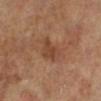Part of a total-body skin-imaging series; this lesion was reviewed on a skin check and was not flagged for biopsy. A 15 mm crop from a total-body photograph taken for skin-cancer surveillance. A female patient aged 63–67. Imaged with cross-polarized lighting. The lesion-visualizer software estimated a lesion area of about 6.5 mm² and a symmetry-axis asymmetry near 0.3. The analysis additionally found a mean CIELAB color near L≈42 a*≈21 b*≈30 and a lesion–skin lightness drop of about 8. And it measured a border-irregularity rating of about 3.5/10, a color-variation rating of about 2.5/10, and peripheral color asymmetry of about 1. Approximately 3.5 mm at its widest. Located on the left leg.